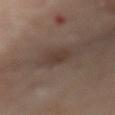{"biopsy_status": "not biopsied; imaged during a skin examination", "image": {"source": "total-body photography crop", "field_of_view_mm": 15}, "site": "back", "lighting": "cross-polarized", "patient": {"sex": "female", "age_approx": 60}, "lesion_size": {"long_diameter_mm_approx": 4.0}}